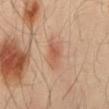Q: Was this lesion biopsied?
A: no biopsy performed (imaged during a skin exam)
Q: Illumination type?
A: cross-polarized illumination
Q: Lesion location?
A: the back
Q: Lesion size?
A: ≈4 mm
Q: What kind of image is this?
A: ~15 mm crop, total-body skin-cancer survey
Q: Automated lesion metrics?
A: border irregularity of about 4 on a 0–10 scale, a within-lesion color-variation index near 2/10, and radial color variation of about 0.5; a classifier nevus-likeness of about 80/100 and a lesion-detection confidence of about 100/100
Q: What are the patient's age and sex?
A: male, in their mid- to late 60s